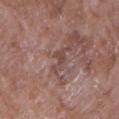- follow-up — no biopsy performed (imaged during a skin exam)